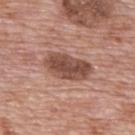Captured during whole-body skin photography for melanoma surveillance; the lesion was not biopsied. The lesion's longest dimension is about 5.5 mm. Cropped from a whole-body photographic skin survey; the tile spans about 15 mm. A male subject, aged around 70. From the upper back.